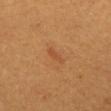Assessment: No biopsy was performed on this lesion — it was imaged during a full skin examination and was not determined to be concerning. Acquisition and patient details: The tile uses cross-polarized illumination. A roughly 15 mm field-of-view crop from a total-body skin photograph. The recorded lesion diameter is about 2.5 mm. The patient is a male approximately 60 years of age. Automated tile analysis of the lesion measured a border-irregularity index near 4.5/10, a color-variation rating of about 0/10, and radial color variation of about 0. The lesion is located on the front of the torso.